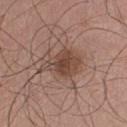Impression: Part of a total-body skin-imaging series; this lesion was reviewed on a skin check and was not flagged for biopsy. Clinical summary: A male subject aged 28 to 32. The lesion is on the chest. Cropped from a whole-body photographic skin survey; the tile spans about 15 mm.